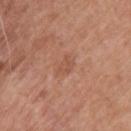| key | value |
|---|---|
| notes | catalogued during a skin exam; not biopsied |
| TBP lesion metrics | a lesion area of about 3.5 mm² and an eccentricity of roughly 0.7; a mean CIELAB color near L≈53 a*≈23 b*≈31 and a normalized border contrast of about 4.5; a border-irregularity rating of about 3.5/10 and peripheral color asymmetry of about 0.5; a nevus-likeness score of about 0/100 and a lesion-detection confidence of about 100/100 |
| image source | total-body-photography crop, ~15 mm field of view |
| lesion diameter | ≈2.5 mm |
| location | the upper back |
| patient | male, in their mid-60s |